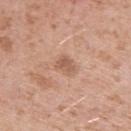biopsy status: no biopsy performed (imaged during a skin exam).